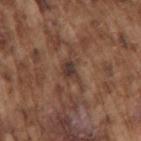Q: Is there a histopathology result?
A: catalogued during a skin exam; not biopsied
Q: Automated lesion metrics?
A: a border-irregularity index near 4.5/10, a within-lesion color-variation index near 4/10, and radial color variation of about 1.5; a classifier nevus-likeness of about 0/100 and lesion-presence confidence of about 75/100
Q: Lesion size?
A: about 3 mm
Q: What is the anatomic site?
A: the right upper arm
Q: What lighting was used for the tile?
A: white-light illumination
Q: What kind of image is this?
A: ~15 mm tile from a whole-body skin photo
Q: What are the patient's age and sex?
A: male, aged around 75